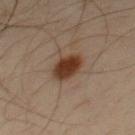Q: Was a biopsy performed?
A: imaged on a skin check; not biopsied
Q: What lighting was used for the tile?
A: cross-polarized illumination
Q: How large is the lesion?
A: ≈3.5 mm
Q: What is the anatomic site?
A: the chest
Q: How was this image acquired?
A: total-body-photography crop, ~15 mm field of view
Q: What did automated image analysis measure?
A: a lesion area of about 9 mm², an outline eccentricity of about 0.5 (0 = round, 1 = elongated), and a shape-asymmetry score of about 0.15 (0 = symmetric); a border-irregularity rating of about 1.5/10, a color-variation rating of about 4.5/10, and a peripheral color-asymmetry measure near 1.5; a nevus-likeness score of about 100/100
Q: What are the patient's age and sex?
A: male, in their 40s